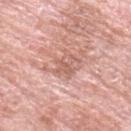biopsy status: no biopsy performed (imaged during a skin exam) | illumination: white-light | body site: the arm | image: total-body-photography crop, ~15 mm field of view | lesion size: ≈3 mm | image-analysis metrics: a lesion color around L≈61 a*≈23 b*≈27 in CIELAB, a lesion–skin lightness drop of about 8, and a lesion-to-skin contrast of about 5.5 (normalized; higher = more distinct); a classifier nevus-likeness of about 0/100 and a lesion-detection confidence of about 85/100 | patient: female, about 60 years old.